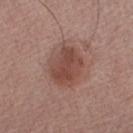The recorded lesion diameter is about 5 mm. A roughly 15 mm field-of-view crop from a total-body skin photograph. The patient is a male aged approximately 55. From the right upper arm. Captured under white-light illumination.